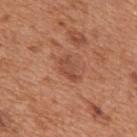notes — catalogued during a skin exam; not biopsied
acquisition — total-body-photography crop, ~15 mm field of view
subject — male, aged approximately 65
diameter — ≈3 mm
location — the mid back